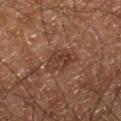Assessment: The lesion was photographed on a routine skin check and not biopsied; there is no pathology result. Clinical summary: On the leg. Measured at roughly 4 mm in maximum diameter. An algorithmic analysis of the crop reported a shape eccentricity near 0.8 and two-axis asymmetry of about 0.2. The software also gave a border-irregularity index near 2.5/10 and internal color variation of about 4 on a 0–10 scale. And it measured a classifier nevus-likeness of about 5/100 and a lesion-detection confidence of about 95/100. A close-up tile cropped from a whole-body skin photograph, about 15 mm across. A male patient, aged 58–62.